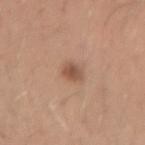Assessment: This lesion was catalogued during total-body skin photography and was not selected for biopsy. Acquisition and patient details: A male subject in their 30s. A 15 mm close-up tile from a total-body photography series done for melanoma screening. The lesion-visualizer software estimated a mean CIELAB color near L≈52 a*≈21 b*≈30, a lesion–skin lightness drop of about 11, and a normalized lesion–skin contrast near 7.5. And it measured a border-irregularity index near 1.5/10 and a color-variation rating of about 4.5/10. The lesion is located on the left forearm.